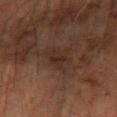Captured during whole-body skin photography for melanoma surveillance; the lesion was not biopsied. On the left forearm. The tile uses cross-polarized illumination. A 15 mm close-up tile from a total-body photography series done for melanoma screening. The subject is a male approximately 70 years of age. The lesion's longest dimension is about 3.5 mm.